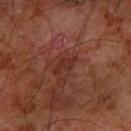Q: Is there a histopathology result?
A: imaged on a skin check; not biopsied
Q: What is the anatomic site?
A: the left forearm
Q: Who is the patient?
A: male, about 60 years old
Q: What is the imaging modality?
A: ~15 mm tile from a whole-body skin photo